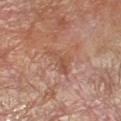biopsy status — catalogued during a skin exam; not biopsied | tile lighting — cross-polarized | diameter — ≈3.5 mm | patient — female, about 55 years old | automated metrics — an area of roughly 6 mm², an eccentricity of roughly 0.7, and a shape-asymmetry score of about 0.55 (0 = symmetric); an automated nevus-likeness rating near 0 out of 100 and lesion-presence confidence of about 100/100 | site — the arm | image — ~15 mm tile from a whole-body skin photo.